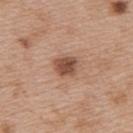  biopsy_status: not biopsied; imaged during a skin examination
  lighting: white-light
  patient:
    sex: female
    age_approx: 40
  lesion_size:
    long_diameter_mm_approx: 2.5
  image:
    source: total-body photography crop
    field_of_view_mm: 15
  site: upper back